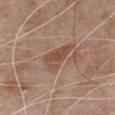Notes:
• illumination: white-light illumination
• image-analysis metrics: a footprint of about 8 mm², a shape eccentricity near 0.8, and a symmetry-axis asymmetry near 0.45; an average lesion color of about L≈49 a*≈20 b*≈29 (CIELAB) and a lesion–skin lightness drop of about 9
• image source: ~15 mm crop, total-body skin-cancer survey
• patient: male, aged around 80
• size: ≈4 mm
• body site: the chest